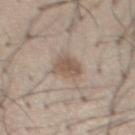| key | value |
|---|---|
| notes | no biopsy performed (imaged during a skin exam) |
| acquisition | total-body-photography crop, ~15 mm field of view |
| tile lighting | white-light |
| location | the chest |
| subject | male, aged approximately 55 |
| size | ≈3.5 mm |